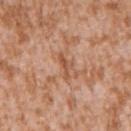From the left upper arm. The patient is a male in their mid-40s. Approximately 3.5 mm at its widest. This image is a 15 mm lesion crop taken from a total-body photograph. This is a white-light tile.